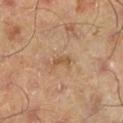Clinical impression:
The lesion was photographed on a routine skin check and not biopsied; there is no pathology result.
Context:
From the left lower leg. A 15 mm close-up tile from a total-body photography series done for melanoma screening. A male subject, in their mid-40s.